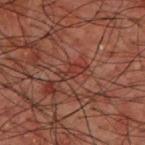{
  "biopsy_status": "not biopsied; imaged during a skin examination",
  "image": {
    "source": "total-body photography crop",
    "field_of_view_mm": 15
  },
  "patient": {
    "sex": "male",
    "age_approx": 50
  },
  "lighting": "cross-polarized",
  "site": "upper back",
  "automated_metrics": {
    "area_mm2_approx": 3.0,
    "eccentricity": 0.9,
    "shape_asymmetry": 0.35,
    "cielab_L": 26,
    "cielab_a": 21,
    "cielab_b": 21,
    "vs_skin_darker_L": 5.0,
    "nevus_likeness_0_100": 0,
    "lesion_detection_confidence_0_100": 75
  }
}